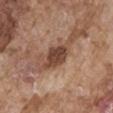Imaged during a routine full-body skin examination; the lesion was not biopsied and no histopathology is available. The subject is a male in their mid- to late 70s. Captured under white-light illumination. On the right upper arm. Approximately 4 mm at its widest. A 15 mm close-up extracted from a 3D total-body photography capture.